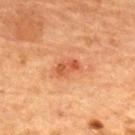Recorded during total-body skin imaging; not selected for excision or biopsy.
A female subject, in their 70s.
From the back.
A 15 mm close-up extracted from a 3D total-body photography capture.
Captured under cross-polarized illumination.